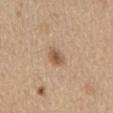{
  "biopsy_status": "not biopsied; imaged during a skin examination",
  "site": "front of the torso",
  "lighting": "white-light",
  "patient": {
    "sex": "male",
    "age_approx": 70
  },
  "image": {
    "source": "total-body photography crop",
    "field_of_view_mm": 15
  },
  "lesion_size": {
    "long_diameter_mm_approx": 3.0
  }
}